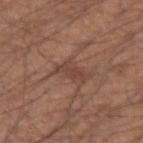Q: What is the anatomic site?
A: the left forearm
Q: What are the patient's age and sex?
A: male, in their mid-30s
Q: What kind of image is this?
A: ~15 mm crop, total-body skin-cancer survey
Q: What is the lesion's diameter?
A: about 3 mm
Q: Automated lesion metrics?
A: border irregularity of about 5 on a 0–10 scale and internal color variation of about 1 on a 0–10 scale
Q: How was the tile lit?
A: white-light